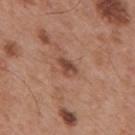{"biopsy_status": "not biopsied; imaged during a skin examination", "site": "mid back", "patient": {"sex": "male", "age_approx": 55}, "image": {"source": "total-body photography crop", "field_of_view_mm": 15}}